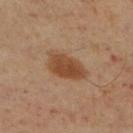The lesion was tiled from a total-body skin photograph and was not biopsied.
The patient is a male roughly 60 years of age.
The total-body-photography lesion software estimated an eccentricity of roughly 0.85 and two-axis asymmetry of about 0.25.
Captured under cross-polarized illumination.
Longest diameter approximately 5.5 mm.
The lesion is on the right lower leg.
Cropped from a total-body skin-imaging series; the visible field is about 15 mm.